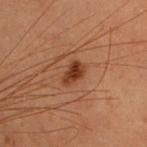acquisition = ~15 mm tile from a whole-body skin photo; subject = female, aged around 40; body site = the upper back.